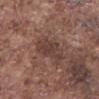Recorded during total-body skin imaging; not selected for excision or biopsy.
A male subject, in their mid- to late 70s.
Cropped from a total-body skin-imaging series; the visible field is about 15 mm.
The lesion is located on the mid back.
The recorded lesion diameter is about 4.5 mm.